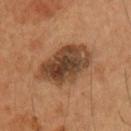Clinical impression: Captured during whole-body skin photography for melanoma surveillance; the lesion was not biopsied. Background: Measured at roughly 6.5 mm in maximum diameter. The total-body-photography lesion software estimated a lesion area of about 21 mm² and an eccentricity of roughly 0.75. The software also gave a lesion–skin lightness drop of about 13 and a lesion-to-skin contrast of about 11 (normalized; higher = more distinct). The software also gave a detector confidence of about 100 out of 100 that the crop contains a lesion. From the left upper arm. A roughly 15 mm field-of-view crop from a total-body skin photograph. A male subject in their mid-50s.